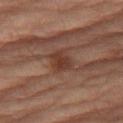Part of a total-body skin-imaging series; this lesion was reviewed on a skin check and was not flagged for biopsy. Located on the left thigh. A male subject, aged 68–72. Automated image analysis of the tile measured a shape eccentricity near 0.7. And it measured an average lesion color of about L≈27 a*≈16 b*≈21 (CIELAB) and roughly 6 lightness units darker than nearby skin. The analysis additionally found a border-irregularity index near 2.5/10 and a color-variation rating of about 2.5/10. It also reported a classifier nevus-likeness of about 5/100 and a lesion-detection confidence of about 100/100. Cropped from a total-body skin-imaging series; the visible field is about 15 mm. The tile uses cross-polarized illumination. Measured at roughly 3 mm in maximum diameter.